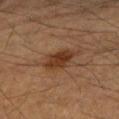Part of a total-body skin-imaging series; this lesion was reviewed on a skin check and was not flagged for biopsy.
The patient is a male in their mid- to late 80s or older.
The total-body-photography lesion software estimated a lesion area of about 7 mm² and two-axis asymmetry of about 0.2. And it measured a color-variation rating of about 3.5/10 and radial color variation of about 1.5. The software also gave lesion-presence confidence of about 100/100.
Imaged with cross-polarized lighting.
Approximately 4 mm at its widest.
A close-up tile cropped from a whole-body skin photograph, about 15 mm across.
Located on the right upper arm.